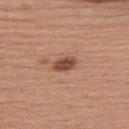No biopsy was performed on this lesion — it was imaged during a full skin examination and was not determined to be concerning. About 3 mm across. The lesion is located on the upper back. The tile uses white-light illumination. Automated tile analysis of the lesion measured an eccentricity of roughly 0.85 and a shape-asymmetry score of about 0.3 (0 = symmetric). The analysis additionally found border irregularity of about 2.5 on a 0–10 scale, a within-lesion color-variation index near 2.5/10, and peripheral color asymmetry of about 1. And it measured an automated nevus-likeness rating near 90 out of 100 and lesion-presence confidence of about 100/100. A male patient in their mid-50s. A 15 mm close-up tile from a total-body photography series done for melanoma screening.